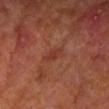<case>
  <lighting>cross-polarized</lighting>
  <automated_metrics>
    <area_mm2_approx>2.0</area_mm2_approx>
    <eccentricity>0.9</eccentricity>
    <shape_asymmetry>0.4</shape_asymmetry>
    <vs_skin_darker_L>6.0</vs_skin_darker_L>
    <vs_skin_contrast_norm>5.5</vs_skin_contrast_norm>
    <peripheral_color_asymmetry>0.0</peripheral_color_asymmetry>
  </automated_metrics>
  <lesion_size>
    <long_diameter_mm_approx>2.5</long_diameter_mm_approx>
  </lesion_size>
  <site>head or neck</site>
  <image>
    <source>total-body photography crop</source>
    <field_of_view_mm>15</field_of_view_mm>
  </image>
  <patient>
    <sex>male</sex>
    <age_approx>65</age_approx>
  </patient>
</case>